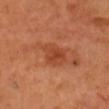Findings:
– image: 15 mm crop, total-body photography
– subject: male, aged around 50
– diameter: ≈4 mm
– location: the head or neck
– lighting: cross-polarized